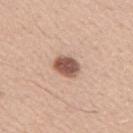Recorded during total-body skin imaging; not selected for excision or biopsy.